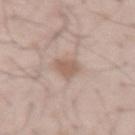workup = catalogued during a skin exam; not biopsied
subject = male, aged around 55
site = the right thigh
acquisition = total-body-photography crop, ~15 mm field of view